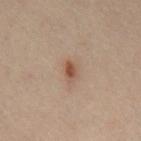Case summary:
– biopsy status · catalogued during a skin exam; not biopsied
– subject · male, aged 48–52
– lesion size · ~2.5 mm (longest diameter)
– location · the chest
– illumination · cross-polarized
– acquisition · total-body-photography crop, ~15 mm field of view